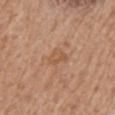Captured during whole-body skin photography for melanoma surveillance; the lesion was not biopsied. The lesion is on the mid back. A roughly 15 mm field-of-view crop from a total-body skin photograph. A male subject roughly 70 years of age. The recorded lesion diameter is about 3 mm.